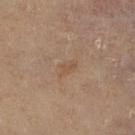Impression: Captured during whole-body skin photography for melanoma surveillance; the lesion was not biopsied. Clinical summary: Located on the right lower leg. The lesion-visualizer software estimated an area of roughly 2.5 mm², an outline eccentricity of about 0.85 (0 = round, 1 = elongated), and a shape-asymmetry score of about 0.3 (0 = symmetric). And it measured an average lesion color of about L≈51 a*≈16 b*≈30 (CIELAB), about 5 CIELAB-L* units darker than the surrounding skin, and a lesion-to-skin contrast of about 5 (normalized; higher = more distinct). The analysis additionally found a classifier nevus-likeness of about 0/100 and lesion-presence confidence of about 100/100. Captured under cross-polarized illumination. A female subject about 60 years old. A lesion tile, about 15 mm wide, cut from a 3D total-body photograph. The lesion's longest dimension is about 2.5 mm.